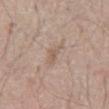biopsy status: catalogued during a skin exam; not biopsied
lighting: white-light
image: ~15 mm crop, total-body skin-cancer survey
subject: male, in their mid-60s
automated lesion analysis: an area of roughly 3.5 mm², an outline eccentricity of about 0.85 (0 = round, 1 = elongated), and a shape-asymmetry score of about 0.5 (0 = symmetric); a lesion color around L≈58 a*≈15 b*≈27 in CIELAB, roughly 7 lightness units darker than nearby skin, and a normalized lesion–skin contrast near 5; border irregularity of about 5 on a 0–10 scale, a within-lesion color-variation index near 1/10, and a peripheral color-asymmetry measure near 0; an automated nevus-likeness rating near 0 out of 100 and a detector confidence of about 100 out of 100 that the crop contains a lesion
site: the abdomen